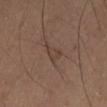Part of a total-body skin-imaging series; this lesion was reviewed on a skin check and was not flagged for biopsy. This is a cross-polarized tile. On the lower back. A 15 mm crop from a total-body photograph taken for skin-cancer surveillance. The patient is a male in their mid-60s.